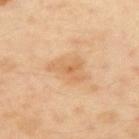{"biopsy_status": "not biopsied; imaged during a skin examination", "patient": {"sex": "male", "age_approx": 50}, "image": {"source": "total-body photography crop", "field_of_view_mm": 15}, "lighting": "cross-polarized", "site": "left upper arm", "automated_metrics": {"area_mm2_approx": 8.5, "eccentricity": 0.75, "cielab_L": 64, "cielab_a": 20, "cielab_b": 39, "vs_skin_darker_L": 8.0, "vs_skin_contrast_norm": 5.5, "border_irregularity_0_10": 4.5, "color_variation_0_10": 3.5, "peripheral_color_asymmetry": 1.0, "nevus_likeness_0_100": 5, "lesion_detection_confidence_0_100": 100}, "lesion_size": {"long_diameter_mm_approx": 4.5}}